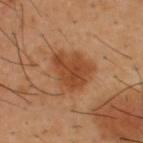Recorded during total-body skin imaging; not selected for excision or biopsy.
Imaged with cross-polarized lighting.
An algorithmic analysis of the crop reported a footprint of about 16 mm² and a symmetry-axis asymmetry near 0.3. The analysis additionally found a lesion–skin lightness drop of about 10 and a lesion-to-skin contrast of about 8 (normalized; higher = more distinct). And it measured border irregularity of about 3 on a 0–10 scale, a within-lesion color-variation index near 3.5/10, and a peripheral color-asymmetry measure near 1.
The lesion is on the upper back.
A male subject, aged 38 to 42.
A 15 mm crop from a total-body photograph taken for skin-cancer surveillance.